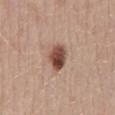Recorded during total-body skin imaging; not selected for excision or biopsy. The patient is a female aged 38 to 42. The lesion is on the abdomen. Approximately 3.5 mm at its widest. Imaged with white-light lighting. A roughly 15 mm field-of-view crop from a total-body skin photograph.